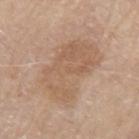<lesion>
  <biopsy_status>not biopsied; imaged during a skin examination</biopsy_status>
  <patient>
    <sex>male</sex>
    <age_approx>80</age_approx>
  </patient>
  <lighting>white-light</lighting>
  <site>right arm</site>
  <image>
    <source>total-body photography crop</source>
    <field_of_view_mm>15</field_of_view_mm>
  </image>
  <automated_metrics>
    <area_mm2_approx>32.0</area_mm2_approx>
    <shape_asymmetry>0.25</shape_asymmetry>
    <cielab_L>59</cielab_L>
    <cielab_a>17</cielab_a>
    <cielab_b>31</cielab_b>
    <vs_skin_darker_L>8.0</vs_skin_darker_L>
    <vs_skin_contrast_norm>5.5</vs_skin_contrast_norm>
  </automated_metrics>
</lesion>